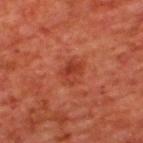Captured during whole-body skin photography for melanoma surveillance; the lesion was not biopsied.
Cropped from a total-body skin-imaging series; the visible field is about 15 mm.
A male patient, approximately 65 years of age.
Automated tile analysis of the lesion measured a lesion area of about 5 mm², an eccentricity of roughly 0.65, and a symmetry-axis asymmetry near 0.3. The analysis additionally found a mean CIELAB color near L≈37 a*≈31 b*≈32 and roughly 7 lightness units darker than nearby skin. And it measured a within-lesion color-variation index near 3/10 and radial color variation of about 1. The software also gave a classifier nevus-likeness of about 45/100 and a detector confidence of about 100 out of 100 that the crop contains a lesion.
Located on the back.